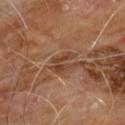Findings:
– workup · catalogued during a skin exam; not biopsied
– body site · the chest
– automated metrics · a lesion area of about 4.5 mm² and an eccentricity of roughly 0.85; a classifier nevus-likeness of about 0/100 and lesion-presence confidence of about 90/100
– patient · male, aged around 60
– image source · total-body-photography crop, ~15 mm field of view
– lesion diameter · ≈3.5 mm
– tile lighting · cross-polarized illumination